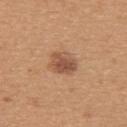Assessment:
The lesion was tiled from a total-body skin photograph and was not biopsied.
Context:
Measured at roughly 3 mm in maximum diameter. A 15 mm crop from a total-body photograph taken for skin-cancer surveillance. On the upper back. A female subject roughly 30 years of age. This is a white-light tile.